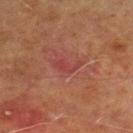notes: no biopsy performed (imaged during a skin exam) | lesion size: about 3 mm | location: the left lower leg | lighting: cross-polarized | image source: total-body-photography crop, ~15 mm field of view | subject: male, about 70 years old | automated lesion analysis: a footprint of about 3.5 mm² and a shape eccentricity near 0.85.